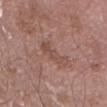follow-up = no biopsy performed (imaged during a skin exam); diameter = ~4 mm (longest diameter); imaging modality = 15 mm crop, total-body photography; lighting = white-light illumination; site = the lower back; patient = male, aged approximately 55.